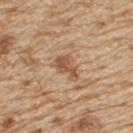follow-up = no biopsy performed (imaged during a skin exam)
tile lighting = white-light illumination
diameter = ~3.5 mm (longest diameter)
subject = male, aged 68 to 72
TBP lesion metrics = a mean CIELAB color near L≈54 a*≈20 b*≈34, roughly 11 lightness units darker than nearby skin, and a normalized lesion–skin contrast near 7.5; a border-irregularity rating of about 4/10 and a peripheral color-asymmetry measure near 1; a detector confidence of about 100 out of 100 that the crop contains a lesion
acquisition = total-body-photography crop, ~15 mm field of view
body site = the back